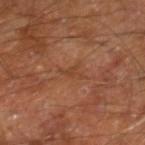{"biopsy_status": "not biopsied; imaged during a skin examination", "automated_metrics": {"border_irregularity_0_10": 5.5, "peripheral_color_asymmetry": 0.0, "nevus_likeness_0_100": 0, "lesion_detection_confidence_0_100": 65}, "site": "right leg", "patient": {"sex": "male", "age_approx": 60}, "image": {"source": "total-body photography crop", "field_of_view_mm": 15}, "lesion_size": {"long_diameter_mm_approx": 3.0}, "lighting": "cross-polarized"}